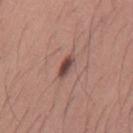Recorded during total-body skin imaging; not selected for excision or biopsy. A 15 mm close-up tile from a total-body photography series done for melanoma screening. The lesion-visualizer software estimated a footprint of about 3.5 mm² and a shape-asymmetry score of about 0.3 (0 = symmetric). It also reported a nevus-likeness score of about 80/100 and a lesion-detection confidence of about 100/100. Measured at roughly 3 mm in maximum diameter. A male patient approximately 35 years of age. From the mid back.